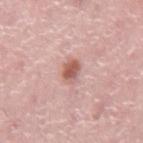Notes:
- biopsy status: catalogued during a skin exam; not biopsied
- patient: male, roughly 75 years of age
- location: the back
- lesion size: ≈2.5 mm
- illumination: white-light
- imaging modality: ~15 mm tile from a whole-body skin photo